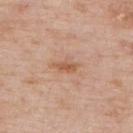Notes:
- image source · ~15 mm tile from a whole-body skin photo
- location · the upper back
- lesion size · ~3 mm (longest diameter)
- patient · male, roughly 75 years of age
- automated lesion analysis · a footprint of about 3.5 mm² and an outline eccentricity of about 0.9 (0 = round, 1 = elongated); a mean CIELAB color near L≈57 a*≈21 b*≈33, about 9 CIELAB-L* units darker than the surrounding skin, and a normalized border contrast of about 7; border irregularity of about 2.5 on a 0–10 scale and a peripheral color-asymmetry measure near 1
- tile lighting · white-light